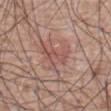Notes:
* biopsy status · total-body-photography surveillance lesion; no biopsy